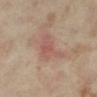biopsy status: total-body-photography surveillance lesion; no biopsy
patient: female, roughly 35 years of age
image source: ~15 mm crop, total-body skin-cancer survey
tile lighting: cross-polarized
location: the right lower leg
diameter: ≈5 mm